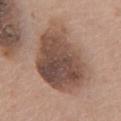Case summary:
– biopsy status: imaged on a skin check; not biopsied
– automated metrics: a footprint of about 41 mm², an eccentricity of roughly 0.6, and a shape-asymmetry score of about 0.25 (0 = symmetric); a border-irregularity index near 2.5/10, internal color variation of about 7 on a 0–10 scale, and radial color variation of about 2; a lesion-detection confidence of about 100/100
– anatomic site: the chest
– imaging modality: 15 mm crop, total-body photography
– size: ≈8.5 mm
– subject: female, in their 60s
– lighting: white-light